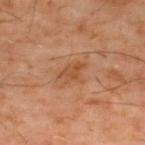| field | value |
|---|---|
| workup | no biopsy performed (imaged during a skin exam) |
| anatomic site | the mid back |
| lighting | cross-polarized |
| image | total-body-photography crop, ~15 mm field of view |
| patient | male, aged around 60 |